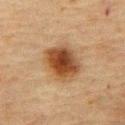Part of a total-body skin-imaging series; this lesion was reviewed on a skin check and was not flagged for biopsy. A male patient, in their mid- to late 70s. The lesion-visualizer software estimated an area of roughly 15 mm², a shape eccentricity near 0.6, and a shape-asymmetry score of about 0.15 (0 = symmetric). It also reported an average lesion color of about L≈38 a*≈19 b*≈31 (CIELAB) and a lesion-to-skin contrast of about 11 (normalized; higher = more distinct). And it measured lesion-presence confidence of about 100/100. Longest diameter approximately 4.5 mm. On the abdomen. Captured under cross-polarized illumination. A close-up tile cropped from a whole-body skin photograph, about 15 mm across.